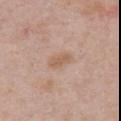{
  "biopsy_status": "not biopsied; imaged during a skin examination",
  "patient": {
    "sex": "male",
    "age_approx": 50
  },
  "site": "chest",
  "image": {
    "source": "total-body photography crop",
    "field_of_view_mm": 15
  },
  "lesion_size": {
    "long_diameter_mm_approx": 3.0
  }
}